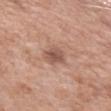Impression:
Imaged during a routine full-body skin examination; the lesion was not biopsied and no histopathology is available.
Acquisition and patient details:
A 15 mm close-up tile from a total-body photography series done for melanoma screening. Captured under white-light illumination. Longest diameter approximately 3 mm. A female patient aged approximately 70. The lesion is located on the left upper arm.